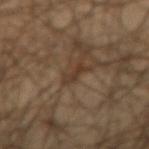<case>
<biopsy_status>not biopsied; imaged during a skin examination</biopsy_status>
<site>left thigh</site>
<image>
  <source>total-body photography crop</source>
  <field_of_view_mm>15</field_of_view_mm>
</image>
<patient>
  <sex>male</sex>
  <age_approx>45</age_approx>
</patient>
<automated_metrics>
  <cielab_L>34</cielab_L>
  <cielab_a>14</cielab_a>
  <cielab_b>26</cielab_b>
  <vs_skin_darker_L>7.0</vs_skin_darker_L>
  <vs_skin_contrast_norm>6.5</vs_skin_contrast_norm>
  <border_irregularity_0_10>4.5</border_irregularity_0_10>
  <color_variation_0_10>0.0</color_variation_0_10>
  <nevus_likeness_0_100>0</nevus_likeness_0_100>
</automated_metrics>
</case>